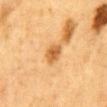<case>
<biopsy_status>not biopsied; imaged during a skin examination</biopsy_status>
<lesion_size>
  <long_diameter_mm_approx>3.0</long_diameter_mm_approx>
</lesion_size>
<image>
  <source>total-body photography crop</source>
  <field_of_view_mm>15</field_of_view_mm>
</image>
<patient>
  <sex>male</sex>
  <age_approx>85+</age_approx>
</patient>
<automated_metrics>
  <eccentricity>0.65</eccentricity>
  <cielab_L>54</cielab_L>
  <cielab_a>21</cielab_a>
  <cielab_b>42</cielab_b>
  <vs_skin_darker_L>11.0</vs_skin_darker_L>
</automated_metrics>
<site>front of the torso</site>
</case>